From the upper back · a male subject in their 80s · cropped from a whole-body photographic skin survey; the tile spans about 15 mm.
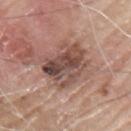Approximately 6 mm at its widest.
The biopsy diagnosis was an invasive melanoma (Breslow thickness 0.5 mm).